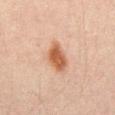<tbp_lesion>
  <biopsy_status>not biopsied; imaged during a skin examination</biopsy_status>
  <image>
    <source>total-body photography crop</source>
    <field_of_view_mm>15</field_of_view_mm>
  </image>
  <patient>
    <sex>male</sex>
    <age_approx>30</age_approx>
  </patient>
  <lighting>cross-polarized</lighting>
  <site>abdomen</site>
  <automated_metrics>
    <area_mm2_approx>7.5</area_mm2_approx>
    <cielab_L>48</cielab_L>
    <cielab_a>21</cielab_a>
    <cielab_b>30</cielab_b>
    <vs_skin_darker_L>12.0</vs_skin_darker_L>
    <vs_skin_contrast_norm>9.5</vs_skin_contrast_norm>
  </automated_metrics>
</tbp_lesion>